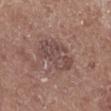Assessment: This lesion was catalogued during total-body skin photography and was not selected for biopsy. Background: The lesion is on the leg. Longest diameter approximately 5 mm. Automated image analysis of the tile measured an average lesion color of about L≈46 a*≈18 b*≈21 (CIELAB) and about 8 CIELAB-L* units darker than the surrounding skin. Captured under white-light illumination. A male patient in their mid- to late 70s. A region of skin cropped from a whole-body photographic capture, roughly 15 mm wide.